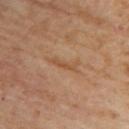biopsy status = catalogued during a skin exam; not biopsied | subject = female, in their 60s | body site = the upper back | imaging modality = total-body-photography crop, ~15 mm field of view.